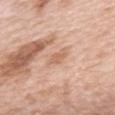Clinical impression:
Imaged during a routine full-body skin examination; the lesion was not biopsied and no histopathology is available.
Clinical summary:
Approximately 2.5 mm at its widest. This image is a 15 mm lesion crop taken from a total-body photograph. A female subject aged 63–67. Located on the upper back. An algorithmic analysis of the crop reported a lesion area of about 3 mm². It also reported border irregularity of about 3 on a 0–10 scale and peripheral color asymmetry of about 0.5.